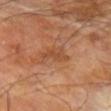{"biopsy_status": "not biopsied; imaged during a skin examination", "lighting": "cross-polarized", "automated_metrics": {"eccentricity": 0.8, "shape_asymmetry": 0.4}, "lesion_size": {"long_diameter_mm_approx": 2.5}, "patient": {"sex": "male", "age_approx": 70}, "image": {"source": "total-body photography crop", "field_of_view_mm": 15}, "site": "left forearm"}